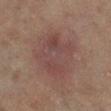Context:
This is a cross-polarized tile. Located on the leg. An algorithmic analysis of the crop reported an area of roughly 26 mm². It also reported a lesion color around L≈37 a*≈16 b*≈18 in CIELAB and a normalized border contrast of about 5.5. It also reported a nevus-likeness score of about 0/100 and a lesion-detection confidence of about 100/100. The recorded lesion diameter is about 6.5 mm. A female subject, aged approximately 80. A roughly 15 mm field-of-view crop from a total-body skin photograph.